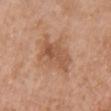| field | value |
|---|---|
| workup | no biopsy performed (imaged during a skin exam) |
| tile lighting | white-light |
| image source | 15 mm crop, total-body photography |
| subject | female, in their 40s |
| diameter | about 5 mm |
| TBP lesion metrics | a footprint of about 13 mm², an eccentricity of roughly 0.65, and a shape-asymmetry score of about 0.25 (0 = symmetric); internal color variation of about 4.5 on a 0–10 scale and peripheral color asymmetry of about 2 |
| location | the chest |